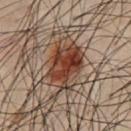{
  "biopsy_status": "not biopsied; imaged during a skin examination",
  "lighting": "cross-polarized",
  "image": {
    "source": "total-body photography crop",
    "field_of_view_mm": 15
  },
  "site": "front of the torso",
  "patient": {
    "sex": "male",
    "age_approx": 50
  }
}